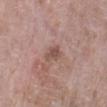This lesion was catalogued during total-body skin photography and was not selected for biopsy.
On the left lower leg.
A lesion tile, about 15 mm wide, cut from a 3D total-body photograph.
A female patient, aged approximately 70.
Approximately 2.5 mm at its widest.
The tile uses white-light illumination.